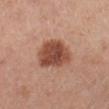| feature | finding |
|---|---|
| workup | no biopsy performed (imaged during a skin exam) |
| automated metrics | a lesion–skin lightness drop of about 14 |
| lighting | cross-polarized |
| body site | the left lower leg |
| lesion size | ≈5 mm |
| imaging modality | ~15 mm tile from a whole-body skin photo |
| subject | male, aged 53–57 |